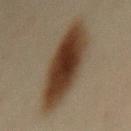biopsy status: imaged on a skin check; not biopsied
image source: total-body-photography crop, ~15 mm field of view
automated metrics: a footprint of about 32 mm², a shape eccentricity near 0.9, and two-axis asymmetry of about 0.15; an average lesion color of about L≈32 a*≈13 b*≈25 (CIELAB) and a normalized lesion–skin contrast near 13; a classifier nevus-likeness of about 100/100
patient: female, in their mid- to late 40s
anatomic site: the back